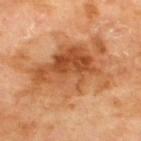{
  "biopsy_status": "not biopsied; imaged during a skin examination",
  "image": {
    "source": "total-body photography crop",
    "field_of_view_mm": 15
  },
  "lesion_size": {
    "long_diameter_mm_approx": 12.0
  },
  "patient": {
    "sex": "male",
    "age_approx": 70
  },
  "site": "upper back",
  "lighting": "cross-polarized"
}